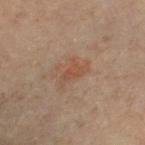The lesion was photographed on a routine skin check and not biopsied; there is no pathology result.